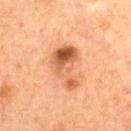Clinical impression: Captured during whole-body skin photography for melanoma surveillance; the lesion was not biopsied. Image and clinical context: Imaged with cross-polarized lighting. Approximately 5.5 mm at its widest. The lesion is on the upper back. The subject is a female in their 60s. A 15 mm close-up extracted from a 3D total-body photography capture. The lesion-visualizer software estimated a shape eccentricity near 0.85. The software also gave a mean CIELAB color near L≈49 a*≈22 b*≈33, roughly 11 lightness units darker than nearby skin, and a normalized lesion–skin contrast near 8. And it measured a within-lesion color-variation index near 9/10 and a peripheral color-asymmetry measure near 3.